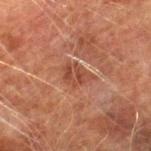<case>
  <biopsy_status>not biopsied; imaged during a skin examination</biopsy_status>
  <site>right lower leg</site>
  <automated_metrics>
    <border_irregularity_0_10>4.0</border_irregularity_0_10>
    <color_variation_0_10>3.0</color_variation_0_10>
  </automated_metrics>
  <patient>
    <sex>male</sex>
    <age_approx>75</age_approx>
  </patient>
  <image>
    <source>total-body photography crop</source>
    <field_of_view_mm>15</field_of_view_mm>
  </image>
  <lighting>cross-polarized</lighting>
  <lesion_size>
    <long_diameter_mm_approx>3.5</long_diameter_mm_approx>
  </lesion_size>
</case>